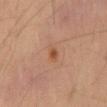notes: total-body-photography surveillance lesion; no biopsy
patient: male, in their mid-50s
body site: the mid back
diameter: ≈3 mm
image source: 15 mm crop, total-body photography